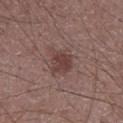| feature | finding |
|---|---|
| biopsy status | catalogued during a skin exam; not biopsied |
| subject | male, approximately 60 years of age |
| illumination | white-light |
| image | ~15 mm crop, total-body skin-cancer survey |
| location | the chest |
| lesion diameter | ~3.5 mm (longest diameter) |